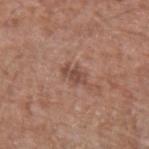Assessment: Part of a total-body skin-imaging series; this lesion was reviewed on a skin check and was not flagged for biopsy. Background: The tile uses white-light illumination. Automated image analysis of the tile measured a footprint of about 3.5 mm², an eccentricity of roughly 0.8, and a shape-asymmetry score of about 0.4 (0 = symmetric). And it measured an average lesion color of about L≈47 a*≈21 b*≈26 (CIELAB), about 9 CIELAB-L* units darker than the surrounding skin, and a normalized border contrast of about 7. The analysis additionally found an automated nevus-likeness rating near 0 out of 100. The lesion is on the arm. A 15 mm crop from a total-body photograph taken for skin-cancer surveillance. The patient is a male aged around 55.